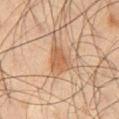Q: Is there a histopathology result?
A: no biopsy performed (imaged during a skin exam)
Q: What is the lesion's diameter?
A: ≈4 mm
Q: Lesion location?
A: the left thigh
Q: How was the tile lit?
A: cross-polarized
Q: What is the imaging modality?
A: ~15 mm crop, total-body skin-cancer survey
Q: Who is the patient?
A: male, aged 43–47